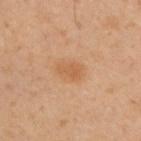Recorded during total-body skin imaging; not selected for excision or biopsy.
From the back.
The subject is a male roughly 45 years of age.
Cropped from a whole-body photographic skin survey; the tile spans about 15 mm.
The lesion's longest dimension is about 3 mm.
This is a cross-polarized tile.